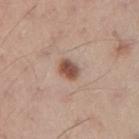follow-up: imaged on a skin check; not biopsied | patient: male, aged 28 to 32 | lighting: white-light illumination | location: the right upper arm | automated lesion analysis: a color-variation rating of about 2.5/10 and radial color variation of about 0.5; a classifier nevus-likeness of about 100/100 | lesion diameter: ≈3 mm | acquisition: ~15 mm tile from a whole-body skin photo.